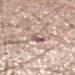This lesion was catalogued during total-body skin photography and was not selected for biopsy. Automated tile analysis of the lesion measured a classifier nevus-likeness of about 0/100 and lesion-presence confidence of about 65/100. Imaged with white-light lighting. A 15 mm close-up tile from a total-body photography series done for melanoma screening. The lesion is located on the mid back. A male subject, aged 58–62.